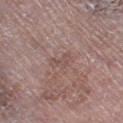Q: Is there a histopathology result?
A: catalogued during a skin exam; not biopsied
Q: Lesion location?
A: the left thigh
Q: How large is the lesion?
A: ≈2.5 mm
Q: What kind of image is this?
A: ~15 mm tile from a whole-body skin photo
Q: Who is the patient?
A: male, aged approximately 80
Q: How was the tile lit?
A: white-light illumination